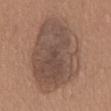No biopsy was performed on this lesion — it was imaged during a full skin examination and was not determined to be concerning.
This image is a 15 mm lesion crop taken from a total-body photograph.
Imaged with white-light lighting.
About 11.5 mm across.
The patient is a male aged 58 to 62.
Automated tile analysis of the lesion measured an eccentricity of roughly 0.85 and two-axis asymmetry of about 0.2. The software also gave a lesion color around L≈48 a*≈16 b*≈25 in CIELAB and a normalized border contrast of about 8. It also reported a within-lesion color-variation index near 4.5/10 and a peripheral color-asymmetry measure near 1.5.
The lesion is on the front of the torso.